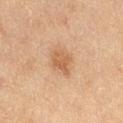Background: Automated tile analysis of the lesion measured an automated nevus-likeness rating near 65 out of 100 and a detector confidence of about 100 out of 100 that the crop contains a lesion. The tile uses cross-polarized illumination. A female subject, aged 58–62. On the leg. A roughly 15 mm field-of-view crop from a total-body skin photograph.